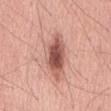Q: What is the anatomic site?
A: the mid back
Q: What is the lesion's diameter?
A: ≈7 mm
Q: Patient demographics?
A: male, approximately 60 years of age
Q: What is the imaging modality?
A: total-body-photography crop, ~15 mm field of view
Q: What lighting was used for the tile?
A: white-light illumination